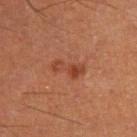{"biopsy_status": "not biopsied; imaged during a skin examination", "site": "right lower leg", "lighting": "cross-polarized", "automated_metrics": {"area_mm2_approx": 5.5, "eccentricity": 0.9, "shape_asymmetry": 0.45, "vs_skin_contrast_norm": 6.5, "nevus_likeness_0_100": 55, "lesion_detection_confidence_0_100": 100}, "lesion_size": {"long_diameter_mm_approx": 4.0}, "patient": {"sex": "male", "age_approx": 60}, "image": {"source": "total-body photography crop", "field_of_view_mm": 15}}